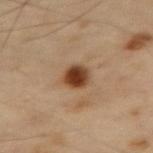Q: Is there a histopathology result?
A: imaged on a skin check; not biopsied